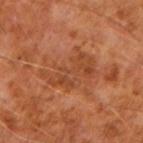| key | value |
|---|---|
| workup | no biopsy performed (imaged during a skin exam) |
| patient | male, aged 58–62 |
| tile lighting | cross-polarized |
| body site | the right upper arm |
| image-analysis metrics | an outline eccentricity of about 0.8 (0 = round, 1 = elongated) and a shape-asymmetry score of about 0.4 (0 = symmetric); border irregularity of about 8 on a 0–10 scale and internal color variation of about 4 on a 0–10 scale; an automated nevus-likeness rating near 0 out of 100 and a detector confidence of about 100 out of 100 that the crop contains a lesion |
| imaging modality | ~15 mm crop, total-body skin-cancer survey |